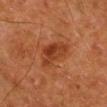Part of a total-body skin-imaging series; this lesion was reviewed on a skin check and was not flagged for biopsy. A male subject roughly 80 years of age. The recorded lesion diameter is about 5 mm. A 15 mm close-up extracted from a 3D total-body photography capture. Automated image analysis of the tile measured a lesion area of about 9.5 mm² and a shape eccentricity near 0.85. And it measured a mean CIELAB color near L≈30 a*≈23 b*≈30 and a lesion-to-skin contrast of about 7.5 (normalized; higher = more distinct). The software also gave a border-irregularity rating of about 3.5/10 and internal color variation of about 4 on a 0–10 scale. It also reported an automated nevus-likeness rating near 25 out of 100 and a lesion-detection confidence of about 100/100. The lesion is on the right lower leg.